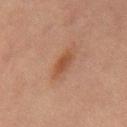Impression:
Part of a total-body skin-imaging series; this lesion was reviewed on a skin check and was not flagged for biopsy.
Image and clinical context:
Cropped from a whole-body photographic skin survey; the tile spans about 15 mm. A male subject, in their mid- to late 60s. On the abdomen.